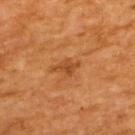Assessment: Part of a total-body skin-imaging series; this lesion was reviewed on a skin check and was not flagged for biopsy. Context: The lesion is on the upper back. The patient is a male approximately 60 years of age. Cropped from a total-body skin-imaging series; the visible field is about 15 mm.